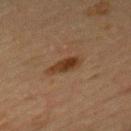| feature | finding |
|---|---|
| workup | no biopsy performed (imaged during a skin exam) |
| location | the back |
| tile lighting | cross-polarized |
| subject | female, aged approximately 60 |
| image-analysis metrics | an average lesion color of about L≈33 a*≈18 b*≈29 (CIELAB) and a normalized border contrast of about 9.5 |
| lesion size | ≈3.5 mm |
| acquisition | total-body-photography crop, ~15 mm field of view |